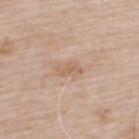{
  "biopsy_status": "not biopsied; imaged during a skin examination",
  "image": {
    "source": "total-body photography crop",
    "field_of_view_mm": 15
  },
  "site": "upper back",
  "patient": {
    "sex": "male",
    "age_approx": 65
  }
}